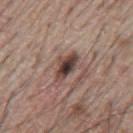notes = no biopsy performed (imaged during a skin exam)
body site = the mid back
automated metrics = an area of roughly 5.5 mm², an eccentricity of roughly 0.85, and two-axis asymmetry of about 0.2; a border-irregularity index near 2.5/10, a within-lesion color-variation index near 7.5/10, and peripheral color asymmetry of about 1.5
acquisition = ~15 mm tile from a whole-body skin photo
subject = male, aged 63 to 67